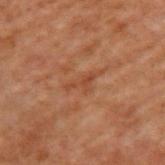This lesion was catalogued during total-body skin photography and was not selected for biopsy. The subject is a male about 70 years old. Imaged with cross-polarized lighting. Automated tile analysis of the lesion measured a normalized border contrast of about 5. The software also gave a border-irregularity rating of about 5.5/10, a within-lesion color-variation index near 2.5/10, and radial color variation of about 1. The recorded lesion diameter is about 4 mm. A region of skin cropped from a whole-body photographic capture, roughly 15 mm wide. The lesion is located on the upper back.